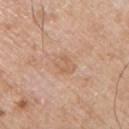The lesion was tiled from a total-body skin photograph and was not biopsied. The tile uses white-light illumination. An algorithmic analysis of the crop reported border irregularity of about 2 on a 0–10 scale, a color-variation rating of about 3/10, and a peripheral color-asymmetry measure near 1. On the left upper arm. A roughly 15 mm field-of-view crop from a total-body skin photograph. A male subject, about 65 years old.